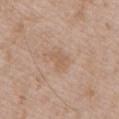automated lesion analysis: an area of roughly 4 mm², a shape eccentricity near 0.7, and a shape-asymmetry score of about 0.3 (0 = symmetric); roughly 6 lightness units darker than nearby skin | lighting: white-light | body site: the chest | image source: 15 mm crop, total-body photography | patient: male, approximately 50 years of age | diameter: about 2.5 mm.